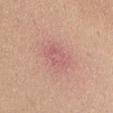Part of a total-body skin-imaging series; this lesion was reviewed on a skin check and was not flagged for biopsy.
Measured at roughly 3.5 mm in maximum diameter.
From the chest.
A male subject, in their mid-30s.
The total-body-photography lesion software estimated a border-irregularity index near 2.5/10 and a color-variation rating of about 2.5/10. The software also gave a classifier nevus-likeness of about 0/100 and a detector confidence of about 100 out of 100 that the crop contains a lesion.
Imaged with white-light lighting.
A close-up tile cropped from a whole-body skin photograph, about 15 mm across.